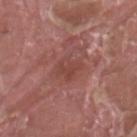| key | value |
|---|---|
| acquisition | 15 mm crop, total-body photography |
| anatomic site | the left thigh |
| patient | male, about 40 years old |